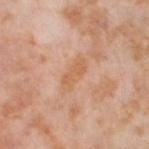workup=catalogued during a skin exam; not biopsied
acquisition=~15 mm tile from a whole-body skin photo
automated metrics=a footprint of about 6 mm², a shape eccentricity near 0.9, and two-axis asymmetry of about 0.25; a border-irregularity rating of about 3/10 and a color-variation rating of about 1.5/10
patient=female, roughly 55 years of age
site=the left thigh